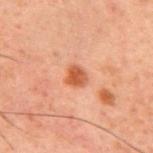  biopsy_status: not biopsied; imaged during a skin examination